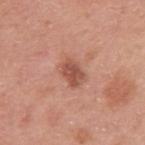  biopsy_status: not biopsied; imaged during a skin examination
  site: right upper arm
  patient:
    sex: male
    age_approx: 50
  image:
    source: total-body photography crop
    field_of_view_mm: 15